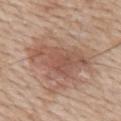Part of a total-body skin-imaging series; this lesion was reviewed on a skin check and was not flagged for biopsy. The lesion-visualizer software estimated an area of roughly 21 mm² and a shape-asymmetry score of about 0.3 (0 = symmetric). And it measured an automated nevus-likeness rating near 40 out of 100 and lesion-presence confidence of about 100/100. A 15 mm close-up tile from a total-body photography series done for melanoma screening. Located on the back. The subject is a male about 60 years old. This is a white-light tile. Measured at roughly 8 mm in maximum diameter.